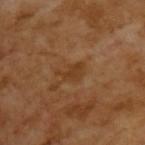A 15 mm close-up tile from a total-body photography series done for melanoma screening.
A male patient, roughly 65 years of age.
About 3 mm across.
Captured under cross-polarized illumination.